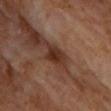Assessment: Recorded during total-body skin imaging; not selected for excision or biopsy. Background: The subject is a male roughly 60 years of age. This is a cross-polarized tile. Automated image analysis of the tile measured an area of roughly 4.5 mm², an outline eccentricity of about 0.75 (0 = round, 1 = elongated), and two-axis asymmetry of about 0.15. The software also gave a lesion color around L≈29 a*≈20 b*≈26 in CIELAB, a lesion–skin lightness drop of about 11, and a lesion-to-skin contrast of about 10.5 (normalized; higher = more distinct). And it measured a nevus-likeness score of about 65/100. This image is a 15 mm lesion crop taken from a total-body photograph. From the chest.